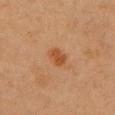Approximately 3 mm at its widest.
From the arm.
A female patient in their 40s.
A roughly 15 mm field-of-view crop from a total-body skin photograph.